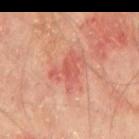Imaged during a routine full-body skin examination; the lesion was not biopsied and no histopathology is available.
A 15 mm close-up tile from a total-body photography series done for melanoma screening.
The lesion is on the mid back.
The tile uses cross-polarized illumination.
A male subject, aged approximately 65.